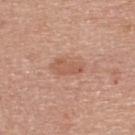Part of a total-body skin-imaging series; this lesion was reviewed on a skin check and was not flagged for biopsy.
This image is a 15 mm lesion crop taken from a total-body photograph.
Located on the upper back.
The patient is a female aged around 65.
This is a white-light tile.
Longest diameter approximately 3.5 mm.